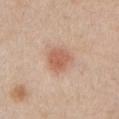Clinical summary: Cropped from a whole-body photographic skin survey; the tile spans about 15 mm. This is a white-light tile. The recorded lesion diameter is about 3 mm. Located on the left upper arm. The patient is a male in their 60s.